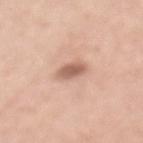<tbp_lesion>
<automated_metrics>
  <area_mm2_approx>4.5</area_mm2_approx>
  <eccentricity>0.85</eccentricity>
  <shape_asymmetry>0.2</shape_asymmetry>
  <cielab_L>60</cielab_L>
  <cielab_a>21</cielab_a>
  <cielab_b>28</cielab_b>
  <vs_skin_darker_L>13.0</vs_skin_darker_L>
  <nevus_likeness_0_100>60</nevus_likeness_0_100>
  <lesion_detection_confidence_0_100>100</lesion_detection_confidence_0_100>
</automated_metrics>
<site>mid back</site>
<lighting>white-light</lighting>
<image>
  <source>total-body photography crop</source>
  <field_of_view_mm>15</field_of_view_mm>
</image>
<lesion_size>
  <long_diameter_mm_approx>3.0</long_diameter_mm_approx>
</lesion_size>
<patient>
  <sex>female</sex>
  <age_approx>50</age_approx>
</patient>
</tbp_lesion>